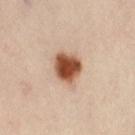{"biopsy_status": "not biopsied; imaged during a skin examination", "patient": {"sex": "female", "age_approx": 40}, "image": {"source": "total-body photography crop", "field_of_view_mm": 15}, "site": "abdomen"}